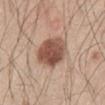The tile uses white-light illumination. About 5 mm across. Automated tile analysis of the lesion measured an area of roughly 14 mm², a shape eccentricity near 0.55, and a symmetry-axis asymmetry near 0.15. The software also gave a lesion color around L≈51 a*≈21 b*≈27 in CIELAB, a lesion–skin lightness drop of about 16, and a normalized border contrast of about 10.5. The analysis additionally found a border-irregularity rating of about 1.5/10, a color-variation rating of about 5/10, and radial color variation of about 2. Cropped from a total-body skin-imaging series; the visible field is about 15 mm. The lesion is located on the left thigh. A male subject in their 60s.